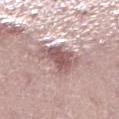{
  "biopsy_status": "not biopsied; imaged during a skin examination",
  "site": "leg",
  "lighting": "white-light",
  "patient": {
    "sex": "female",
    "age_approx": 40
  },
  "image": {
    "source": "total-body photography crop",
    "field_of_view_mm": 15
  },
  "automated_metrics": {
    "area_mm2_approx": 13.0,
    "eccentricity": 0.7,
    "shape_asymmetry": 0.45,
    "nevus_likeness_0_100": 5,
    "lesion_detection_confidence_0_100": 75
  },
  "lesion_size": {
    "long_diameter_mm_approx": 4.5
  }
}